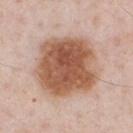- biopsy status · catalogued during a skin exam; not biopsied